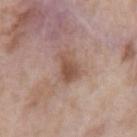Acquisition and patient details: A lesion tile, about 15 mm wide, cut from a 3D total-body photograph. On the left upper arm. A male patient about 60 years old.